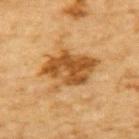Background: A male subject, aged 83 or over. On the upper back. The lesion-visualizer software estimated a footprint of about 19 mm², a shape eccentricity near 0.75, and a shape-asymmetry score of about 0.25 (0 = symmetric). It also reported a lesion-to-skin contrast of about 9.5 (normalized; higher = more distinct). The analysis additionally found a border-irregularity rating of about 4/10 and a peripheral color-asymmetry measure near 2. The software also gave lesion-presence confidence of about 100/100. The lesion's longest dimension is about 6.5 mm. Cropped from a whole-body photographic skin survey; the tile spans about 15 mm.